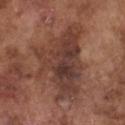<case>
  <biopsy_status>not biopsied; imaged during a skin examination</biopsy_status>
  <lighting>white-light</lighting>
  <patient>
    <sex>male</sex>
    <age_approx>75</age_approx>
  </patient>
  <site>chest</site>
  <automated_metrics>
    <cielab_L>38</cielab_L>
    <cielab_a>20</cielab_a>
    <cielab_b>24</cielab_b>
    <vs_skin_darker_L>10.0</vs_skin_darker_L>
    <vs_skin_contrast_norm>8.5</vs_skin_contrast_norm>
    <border_irregularity_0_10>7.5</border_irregularity_0_10>
    <color_variation_0_10>6.0</color_variation_0_10>
    <peripheral_color_asymmetry>2.0</peripheral_color_asymmetry>
    <nevus_likeness_0_100>0</nevus_likeness_0_100>
    <lesion_detection_confidence_0_100>95</lesion_detection_confidence_0_100>
  </automated_metrics>
  <lesion_size>
    <long_diameter_mm_approx>9.0</long_diameter_mm_approx>
  </lesion_size>
  <image>
    <source>total-body photography crop</source>
    <field_of_view_mm>15</field_of_view_mm>
  </image>
</case>